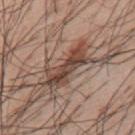Impression:
Part of a total-body skin-imaging series; this lesion was reviewed on a skin check and was not flagged for biopsy.
Image and clinical context:
The lesion is on the upper back. Measured at roughly 7 mm in maximum diameter. Automated image analysis of the tile measured a footprint of about 14 mm² and two-axis asymmetry of about 0.4. The software also gave an average lesion color of about L≈45 a*≈18 b*≈25 (CIELAB), about 13 CIELAB-L* units darker than the surrounding skin, and a normalized border contrast of about 9.5. A male patient, roughly 55 years of age. A 15 mm crop from a total-body photograph taken for skin-cancer surveillance. This is a white-light tile.